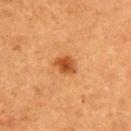Part of a total-body skin-imaging series; this lesion was reviewed on a skin check and was not flagged for biopsy.
A lesion tile, about 15 mm wide, cut from a 3D total-body photograph.
This is a cross-polarized tile.
Longest diameter approximately 3 mm.
Located on the upper back.
A male patient, roughly 50 years of age.
The total-body-photography lesion software estimated a shape eccentricity near 0.65 and a shape-asymmetry score of about 0.25 (0 = symmetric). The software also gave lesion-presence confidence of about 100/100.